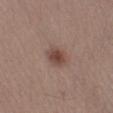follow-up = no biopsy performed (imaged during a skin exam) | acquisition = ~15 mm tile from a whole-body skin photo | subject = male, approximately 40 years of age | location = the leg.